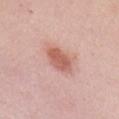Q: What are the patient's age and sex?
A: female, in their mid-30s
Q: Where on the body is the lesion?
A: the chest
Q: How was this image acquired?
A: ~15 mm crop, total-body skin-cancer survey